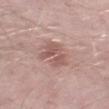{"biopsy_status": "not biopsied; imaged during a skin examination", "patient": {"sex": "male", "age_approx": 50}, "lighting": "white-light", "lesion_size": {"long_diameter_mm_approx": 4.0}, "image": {"source": "total-body photography crop", "field_of_view_mm": 15}, "site": "leg"}